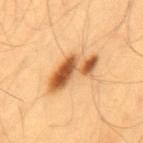Assessment:
Part of a total-body skin-imaging series; this lesion was reviewed on a skin check and was not flagged for biopsy.
Clinical summary:
A 15 mm close-up extracted from a 3D total-body photography capture. A male patient about 55 years old. Imaged with cross-polarized lighting. From the back.